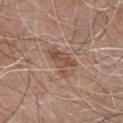follow-up: catalogued during a skin exam; not biopsied | size: ≈4 mm | subject: male, aged approximately 80 | automated metrics: a lesion area of about 7.5 mm², a shape eccentricity near 0.8, and a symmetry-axis asymmetry near 0.5; a border-irregularity index near 6.5/10 and a color-variation rating of about 3.5/10; a lesion-detection confidence of about 100/100 | location: the chest | acquisition: total-body-photography crop, ~15 mm field of view.